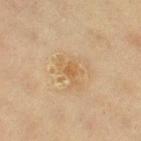Assessment: Captured during whole-body skin photography for melanoma surveillance; the lesion was not biopsied. Clinical summary: A 15 mm close-up extracted from a 3D total-body photography capture. About 3.5 mm across. Captured under cross-polarized illumination. A female subject, roughly 65 years of age. Automated tile analysis of the lesion measured a lesion–skin lightness drop of about 6 and a normalized border contrast of about 5.5. The software also gave border irregularity of about 5.5 on a 0–10 scale, a color-variation rating of about 2/10, and peripheral color asymmetry of about 0.5. It also reported an automated nevus-likeness rating near 0 out of 100 and a lesion-detection confidence of about 100/100. On the right thigh.